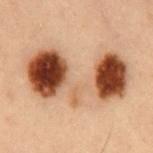Assessment:
The lesion was photographed on a routine skin check and not biopsied; there is no pathology result.
Context:
The recorded lesion diameter is about 11 mm. Located on the chest. A roughly 15 mm field-of-view crop from a total-body skin photograph. This is a cross-polarized tile. The lesion-visualizer software estimated a color-variation rating of about 10/10 and a peripheral color-asymmetry measure near 5.5. It also reported a nevus-likeness score of about 100/100 and a detector confidence of about 100 out of 100 that the crop contains a lesion. A male subject aged around 55.